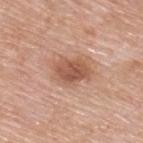| key | value |
|---|---|
| workup | total-body-photography surveillance lesion; no biopsy |
| imaging modality | total-body-photography crop, ~15 mm field of view |
| patient | male, aged 78–82 |
| illumination | white-light |
| size | about 4.5 mm |
| body site | the upper back |